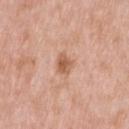{
  "biopsy_status": "not biopsied; imaged during a skin examination",
  "automated_metrics": {
    "vs_skin_darker_L": 10.0,
    "vs_skin_contrast_norm": 7.5,
    "color_variation_0_10": 3.0,
    "lesion_detection_confidence_0_100": 100
  },
  "patient": {
    "sex": "male",
    "age_approx": 50
  },
  "image": {
    "source": "total-body photography crop",
    "field_of_view_mm": 15
  },
  "lesion_size": {
    "long_diameter_mm_approx": 3.0
  },
  "lighting": "white-light",
  "site": "left upper arm"
}